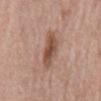biopsy status = no biopsy performed (imaged during a skin exam); patient = male, about 80 years old; automated lesion analysis = a mean CIELAB color near L≈52 a*≈20 b*≈27, a lesion–skin lightness drop of about 11, and a normalized border contrast of about 8; lighting = white-light illumination; imaging modality = ~15 mm crop, total-body skin-cancer survey; anatomic site = the mid back.